The patient is a male roughly 55 years of age. The lesion is on the right lower leg. A 15 mm close-up extracted from a 3D total-body photography capture: 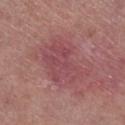The lesion's longest dimension is about 6 mm. The tile uses white-light illumination. The biopsy diagnosis was a nodular basal cell carcinoma — a malignant lesion.